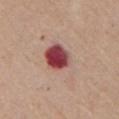Findings:
– workup: no biopsy performed (imaged during a skin exam)
– illumination: white-light
– acquisition: ~15 mm tile from a whole-body skin photo
– diameter: ~5 mm (longest diameter)
– location: the front of the torso
– subject: male, aged approximately 65